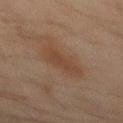{"biopsy_status": "not biopsied; imaged during a skin examination", "patient": {"sex": "male", "age_approx": 45}, "image": {"source": "total-body photography crop", "field_of_view_mm": 15}, "automated_metrics": {"cielab_L": 34, "cielab_a": 15, "cielab_b": 24, "vs_skin_darker_L": 6.0, "vs_skin_contrast_norm": 6.0, "nevus_likeness_0_100": 20}, "site": "mid back"}